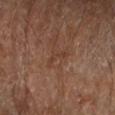{"biopsy_status": "not biopsied; imaged during a skin examination", "image": {"source": "total-body photography crop", "field_of_view_mm": 15}, "site": "right forearm", "automated_metrics": {"area_mm2_approx": 2.5, "eccentricity": 0.9, "shape_asymmetry": 0.55, "border_irregularity_0_10": 6.0, "color_variation_0_10": 0.0, "peripheral_color_asymmetry": 0.0, "lesion_detection_confidence_0_100": 100}, "patient": {"sex": "male", "age_approx": "85+"}, "lighting": "cross-polarized", "lesion_size": {"long_diameter_mm_approx": 3.0}}